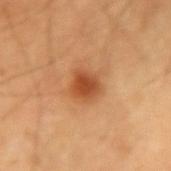The lesion was photographed on a routine skin check and not biopsied; there is no pathology result. The subject is a male aged 58–62. Longest diameter approximately 3 mm. Captured under cross-polarized illumination. Located on the right upper arm. A close-up tile cropped from a whole-body skin photograph, about 15 mm across.